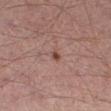* biopsy status: imaged on a skin check; not biopsied
* image: ~15 mm tile from a whole-body skin photo
* tile lighting: cross-polarized illumination
* patient: male, in their 30s
* site: the leg
* diameter: about 1.5 mm